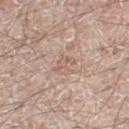Imaged during a routine full-body skin examination; the lesion was not biopsied and no histopathology is available.
A region of skin cropped from a whole-body photographic capture, roughly 15 mm wide.
A male patient, roughly 60 years of age.
On the leg.
The lesion's longest dimension is about 2.5 mm.